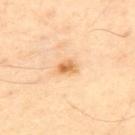biopsy status — imaged on a skin check; not biopsied
diameter — ≈2.5 mm
location — the upper back
subject — male, in their mid- to late 50s
acquisition — total-body-photography crop, ~15 mm field of view
illumination — cross-polarized
automated lesion analysis — a lesion area of about 4 mm², an outline eccentricity of about 0.7 (0 = round, 1 = elongated), and two-axis asymmetry of about 0.2; about 12 CIELAB-L* units darker than the surrounding skin and a normalized border contrast of about 8; a border-irregularity index near 2/10, a within-lesion color-variation index near 4.5/10, and a peripheral color-asymmetry measure near 1.5; a nevus-likeness score of about 65/100 and a detector confidence of about 100 out of 100 that the crop contains a lesion